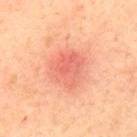The lesion was photographed on a routine skin check and not biopsied; there is no pathology result.
Imaged with cross-polarized lighting.
From the upper back.
Cropped from a total-body skin-imaging series; the visible field is about 15 mm.
A male patient approximately 40 years of age.